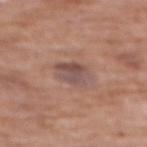Impression: The lesion was photographed on a routine skin check and not biopsied; there is no pathology result. Acquisition and patient details: Located on the upper back. A female subject, about 75 years old. Captured under white-light illumination. Longest diameter approximately 3.5 mm. Automated image analysis of the tile measured a footprint of about 8.5 mm², an eccentricity of roughly 0.55, and a shape-asymmetry score of about 0.25 (0 = symmetric). The software also gave an automated nevus-likeness rating near 0 out of 100 and lesion-presence confidence of about 100/100. A lesion tile, about 15 mm wide, cut from a 3D total-body photograph.